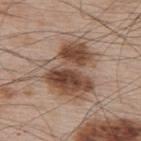Q: Was this lesion biopsied?
A: no biopsy performed (imaged during a skin exam)
Q: Patient demographics?
A: male, aged around 65
Q: Where on the body is the lesion?
A: the upper back
Q: Illumination type?
A: white-light
Q: What is the lesion's diameter?
A: ~7 mm (longest diameter)
Q: What kind of image is this?
A: total-body-photography crop, ~15 mm field of view